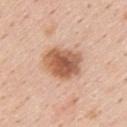Assessment:
The lesion was tiled from a total-body skin photograph and was not biopsied.
Context:
Captured under white-light illumination. Cropped from a total-body skin-imaging series; the visible field is about 15 mm. The subject is a male aged 58 to 62. Approximately 4.5 mm at its widest. The lesion is located on the back.